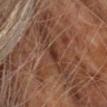{
  "biopsy_status": "not biopsied; imaged during a skin examination",
  "lighting": "cross-polarized",
  "patient": {
    "sex": "male",
    "age_approx": 60
  },
  "site": "chest",
  "lesion_size": {
    "long_diameter_mm_approx": 2.5
  },
  "automated_metrics": {
    "eccentricity": 0.9,
    "shape_asymmetry": 0.35,
    "cielab_L": 32,
    "cielab_a": 21,
    "cielab_b": 26,
    "vs_skin_contrast_norm": 8.5,
    "border_irregularity_0_10": 3.5,
    "color_variation_0_10": 1.0,
    "peripheral_color_asymmetry": 0.5
  },
  "image": {
    "source": "total-body photography crop",
    "field_of_view_mm": 15
  }
}